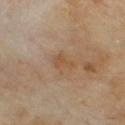The lesion was photographed on a routine skin check and not biopsied; there is no pathology result. A lesion tile, about 15 mm wide, cut from a 3D total-body photograph. Longest diameter approximately 2.5 mm. The tile uses cross-polarized illumination. A male patient, aged 68–72. The lesion is on the chest.